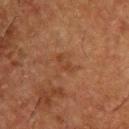automated metrics: an outline eccentricity of about 0.9 (0 = round, 1 = elongated) and a shape-asymmetry score of about 0.5 (0 = symmetric); a mean CIELAB color near L≈32 a*≈19 b*≈27, a lesion–skin lightness drop of about 5, and a normalized border contrast of about 5 | patient: male, approximately 50 years of age | acquisition: ~15 mm tile from a whole-body skin photo | lesion size: ≈2.5 mm | anatomic site: the upper back | lighting: cross-polarized.